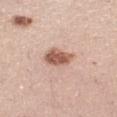Part of a total-body skin-imaging series; this lesion was reviewed on a skin check and was not flagged for biopsy. A female patient aged 38 to 42. A lesion tile, about 15 mm wide, cut from a 3D total-body photograph. About 3 mm across. On the leg. This is a white-light tile.